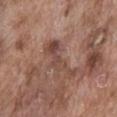workup — catalogued during a skin exam; not biopsied
imaging modality — total-body-photography crop, ~15 mm field of view
tile lighting — white-light illumination
body site — the front of the torso
diameter — about 5.5 mm
subject — male, aged 73–77